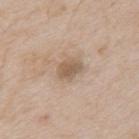Q: Is there a histopathology result?
A: imaged on a skin check; not biopsied
Q: Patient demographics?
A: male, in their mid-60s
Q: Where on the body is the lesion?
A: the back
Q: How was this image acquired?
A: ~15 mm tile from a whole-body skin photo